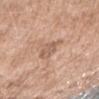Q: How large is the lesion?
A: about 3.5 mm
Q: Where on the body is the lesion?
A: the right forearm
Q: Patient demographics?
A: female, approximately 75 years of age
Q: How was this image acquired?
A: ~15 mm tile from a whole-body skin photo
Q: Illumination type?
A: white-light illumination